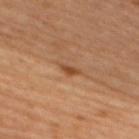workup: imaged on a skin check; not biopsied | acquisition: ~15 mm crop, total-body skin-cancer survey | location: the right upper arm | tile lighting: cross-polarized illumination | patient: female, roughly 45 years of age | lesion size: ~2.5 mm (longest diameter).